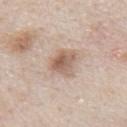Recorded during total-body skin imaging; not selected for excision or biopsy.
A male subject aged 63–67.
Approximately 3.5 mm at its widest.
On the mid back.
A roughly 15 mm field-of-view crop from a total-body skin photograph.
Imaged with white-light lighting.
An algorithmic analysis of the crop reported an eccentricity of roughly 0.6 and two-axis asymmetry of about 0.3. The software also gave a border-irregularity index near 2.5/10, internal color variation of about 6 on a 0–10 scale, and radial color variation of about 2.